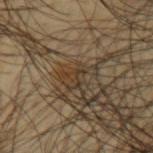notes: no biopsy performed (imaged during a skin exam) | illumination: cross-polarized | subject: male, aged approximately 45 | automated lesion analysis: a lesion area of about 5.5 mm² and a shape-asymmetry score of about 0.6 (0 = symmetric); roughly 7 lightness units darker than nearby skin and a normalized lesion–skin contrast near 7; an automated nevus-likeness rating near 30 out of 100 | anatomic site: the left upper arm | lesion diameter: about 4 mm | acquisition: ~15 mm tile from a whole-body skin photo.